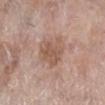| feature | finding |
|---|---|
| workup | no biopsy performed (imaged during a skin exam) |
| image | 15 mm crop, total-body photography |
| subject | female, in their mid-60s |
| illumination | white-light illumination |
| anatomic site | the leg |